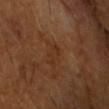Recorded during total-body skin imaging; not selected for excision or biopsy. A male subject, approximately 70 years of age. About 3 mm across. An algorithmic analysis of the crop reported a lesion color around L≈30 a*≈20 b*≈31 in CIELAB, a lesion–skin lightness drop of about 4, and a normalized border contrast of about 4.5. The analysis additionally found border irregularity of about 4.5 on a 0–10 scale, a color-variation rating of about 0.5/10, and peripheral color asymmetry of about 0. And it measured a classifier nevus-likeness of about 0/100 and lesion-presence confidence of about 95/100. The lesion is located on the head or neck. This image is a 15 mm lesion crop taken from a total-body photograph. Imaged with cross-polarized lighting.